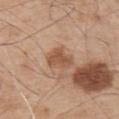<lesion>
<biopsy_status>not biopsied; imaged during a skin examination</biopsy_status>
<patient>
  <sex>male</sex>
  <age_approx>55</age_approx>
</patient>
<lesion_size>
  <long_diameter_mm_approx>3.5</long_diameter_mm_approx>
</lesion_size>
<lighting>white-light</lighting>
<image>
  <source>total-body photography crop</source>
  <field_of_view_mm>15</field_of_view_mm>
</image>
<site>upper back</site>
</lesion>